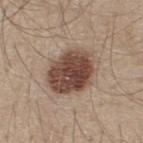• follow-up: no biopsy performed (imaged during a skin exam)
• image: ~15 mm tile from a whole-body skin photo
• location: the upper back
• patient: male, in their 30s
• lighting: white-light illumination
• automated lesion analysis: a footprint of about 21 mm², an eccentricity of roughly 0.7, and a symmetry-axis asymmetry near 0.15; border irregularity of about 2 on a 0–10 scale, a within-lesion color-variation index near 6/10, and peripheral color asymmetry of about 2
• diameter: ≈6 mm